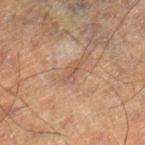biopsy_status: not biopsied; imaged during a skin examination
image:
  source: total-body photography crop
  field_of_view_mm: 15
site: right lower leg
lesion_size:
  long_diameter_mm_approx: 2.5
patient:
  sex: male
  age_approx: 50
automated_metrics:
  cielab_L: 41
  cielab_a: 16
  cielab_b: 23
  vs_skin_darker_L: 6.0
  vs_skin_contrast_norm: 5.0
  border_irregularity_0_10: 6.0
  color_variation_0_10: 0.0